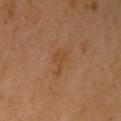Context: A female subject, in their 60s. The lesion is on the left upper arm. A region of skin cropped from a whole-body photographic capture, roughly 15 mm wide. The recorded lesion diameter is about 3.5 mm. Imaged with cross-polarized lighting.